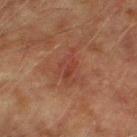* follow-up — total-body-photography surveillance lesion; no biopsy